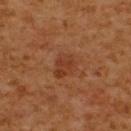| key | value |
|---|---|
| automated metrics | a mean CIELAB color near L≈31 a*≈21 b*≈28, roughly 6 lightness units darker than nearby skin, and a normalized lesion–skin contrast near 6; a border-irregularity index near 2/10, a within-lesion color-variation index near 2.5/10, and a peripheral color-asymmetry measure near 1; a detector confidence of about 100 out of 100 that the crop contains a lesion |
| subject | male, roughly 60 years of age |
| diameter | ~3.5 mm (longest diameter) |
| anatomic site | the upper back |
| imaging modality | total-body-photography crop, ~15 mm field of view |